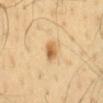{"lighting": "cross-polarized", "site": "back", "patient": {"sex": "male", "age_approx": 65}, "image": {"source": "total-body photography crop", "field_of_view_mm": 15}}